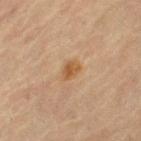– follow-up: catalogued during a skin exam; not biopsied
– illumination: cross-polarized illumination
– anatomic site: the right thigh
– subject: female, roughly 80 years of age
– image: total-body-photography crop, ~15 mm field of view
– lesion diameter: ≈2.5 mm